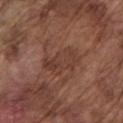Impression: Part of a total-body skin-imaging series; this lesion was reviewed on a skin check and was not flagged for biopsy. Clinical summary: Longest diameter approximately 4.5 mm. The lesion is on the left upper arm. A male patient, approximately 75 years of age. A 15 mm close-up extracted from a 3D total-body photography capture. This is a white-light tile. The lesion-visualizer software estimated an automated nevus-likeness rating near 0 out of 100 and a lesion-detection confidence of about 100/100.